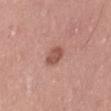This lesion was catalogued during total-body skin photography and was not selected for biopsy.
The lesion is located on the lower back.
A female subject roughly 40 years of age.
Measured at roughly 3 mm in maximum diameter.
Cropped from a total-body skin-imaging series; the visible field is about 15 mm.